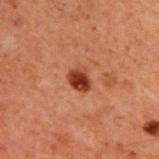Impression:
No biopsy was performed on this lesion — it was imaged during a full skin examination and was not determined to be concerning.
Image and clinical context:
The lesion is located on the upper back. A 15 mm close-up tile from a total-body photography series done for melanoma screening. A male subject in their 60s. This is a cross-polarized tile.